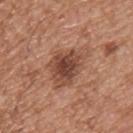biopsy_status: not biopsied; imaged during a skin examination
lesion_size:
  long_diameter_mm_approx: 6.5
patient:
  sex: male
  age_approx: 55
lighting: white-light
site: back
image:
  source: total-body photography crop
  field_of_view_mm: 15